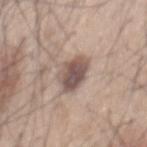The lesion was photographed on a routine skin check and not biopsied; there is no pathology result.
The recorded lesion diameter is about 4 mm.
A male subject, aged 43–47.
Captured under white-light illumination.
Cropped from a whole-body photographic skin survey; the tile spans about 15 mm.
From the mid back.
The total-body-photography lesion software estimated a footprint of about 9 mm², an outline eccentricity of about 0.8 (0 = round, 1 = elongated), and a symmetry-axis asymmetry near 0.2. It also reported a mean CIELAB color near L≈52 a*≈15 b*≈21, roughly 14 lightness units darker than nearby skin, and a normalized border contrast of about 9.5. The software also gave a border-irregularity index near 2.5/10, a color-variation rating of about 5/10, and a peripheral color-asymmetry measure near 1.5.